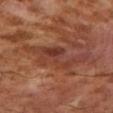Acquisition and patient details: A male subject, aged around 55. This image is a 15 mm lesion crop taken from a total-body photograph. This is a cross-polarized tile. The lesion is on the right upper arm. Automated tile analysis of the lesion measured an average lesion color of about L≈40 a*≈25 b*≈29 (CIELAB), a lesion–skin lightness drop of about 7, and a lesion-to-skin contrast of about 6 (normalized; higher = more distinct). The analysis additionally found a classifier nevus-likeness of about 0/100 and a detector confidence of about 70 out of 100 that the crop contains a lesion.